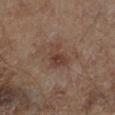Q: Was a biopsy performed?
A: catalogued during a skin exam; not biopsied
Q: What is the lesion's diameter?
A: ≈3.5 mm
Q: Illumination type?
A: cross-polarized
Q: What did automated image analysis measure?
A: an average lesion color of about L≈37 a*≈17 b*≈23 (CIELAB), about 7 CIELAB-L* units darker than the surrounding skin, and a normalized lesion–skin contrast near 6.5
Q: What is the anatomic site?
A: the left lower leg
Q: How was this image acquired?
A: 15 mm crop, total-body photography
Q: Who is the patient?
A: male, aged 63 to 67